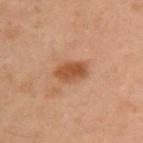<tbp_lesion>
  <biopsy_status>not biopsied; imaged during a skin examination</biopsy_status>
  <patient>
    <sex>female</sex>
    <age_approx>55</age_approx>
  </patient>
  <lesion_size>
    <long_diameter_mm_approx>3.5</long_diameter_mm_approx>
  </lesion_size>
  <site>left arm</site>
  <image>
    <source>total-body photography crop</source>
    <field_of_view_mm>15</field_of_view_mm>
  </image>
</tbp_lesion>